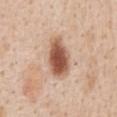Clinical impression:
The lesion was tiled from a total-body skin photograph and was not biopsied.
Image and clinical context:
Approximately 5 mm at its widest. The lesion is on the abdomen. A roughly 15 mm field-of-view crop from a total-body skin photograph. A male subject, roughly 60 years of age.